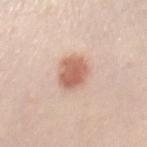Impression: Captured during whole-body skin photography for melanoma surveillance; the lesion was not biopsied. Image and clinical context: A lesion tile, about 15 mm wide, cut from a 3D total-body photograph. A female patient aged around 50. About 4 mm across. The total-body-photography lesion software estimated a lesion area of about 9 mm². The software also gave border irregularity of about 1.5 on a 0–10 scale and peripheral color asymmetry of about 1. The lesion is located on the chest.